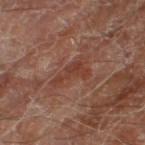{
  "biopsy_status": "not biopsied; imaged during a skin examination",
  "lesion_size": {
    "long_diameter_mm_approx": 4.5
  },
  "lighting": "cross-polarized",
  "patient": {
    "sex": "male",
    "age_approx": 70
  },
  "automated_metrics": {
    "area_mm2_approx": 5.0,
    "eccentricity": 0.9,
    "shape_asymmetry": 0.65,
    "border_irregularity_0_10": 7.5,
    "color_variation_0_10": 1.5,
    "peripheral_color_asymmetry": 0.5,
    "nevus_likeness_0_100": 0,
    "lesion_detection_confidence_0_100": 75
  },
  "site": "leg",
  "image": {
    "source": "total-body photography crop",
    "field_of_view_mm": 15
  }
}